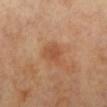{"patient": {"sex": "female", "age_approx": 65}, "image": {"source": "total-body photography crop", "field_of_view_mm": 15}, "automated_metrics": {"color_variation_0_10": 1.5}, "site": "right leg"}